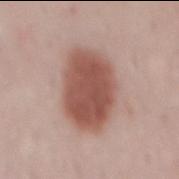Findings:
– follow-up: total-body-photography surveillance lesion; no biopsy
– image source: total-body-photography crop, ~15 mm field of view
– body site: the back
– automated metrics: an area of roughly 27 mm², an outline eccentricity of about 0.75 (0 = round, 1 = elongated), and a shape-asymmetry score of about 0.15 (0 = symmetric)
– tile lighting: white-light
– patient: female, aged around 50